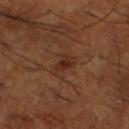biopsy_status: not biopsied; imaged during a skin examination
image:
  source: total-body photography crop
  field_of_view_mm: 15
patient:
  sex: male
  age_approx: 65
site: left lower leg